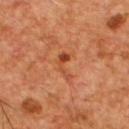{
  "automated_metrics": {
    "area_mm2_approx": 3.5,
    "eccentricity": 0.9,
    "shape_asymmetry": 0.65,
    "cielab_L": 44,
    "cielab_a": 29,
    "cielab_b": 36,
    "vs_skin_darker_L": 9.0,
    "vs_skin_contrast_norm": 7.0,
    "color_variation_0_10": 1.0,
    "peripheral_color_asymmetry": 0.5
  },
  "image": {
    "source": "total-body photography crop",
    "field_of_view_mm": 15
  },
  "patient": {
    "sex": "male",
    "age_approx": 55
  },
  "lighting": "cross-polarized",
  "site": "chest",
  "lesion_size": {
    "long_diameter_mm_approx": 3.5
  }
}